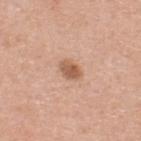| key | value |
|---|---|
| workup | no biopsy performed (imaged during a skin exam) |
| site | the upper back |
| imaging modality | ~15 mm tile from a whole-body skin photo |
| illumination | white-light illumination |
| subject | female, in their 30s |